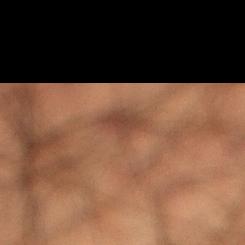Recorded during total-body skin imaging; not selected for excision or biopsy.
The patient is a male aged 48 to 52.
Imaged with cross-polarized lighting.
The lesion's longest dimension is about 3 mm.
Cropped from a whole-body photographic skin survey; the tile spans about 15 mm.
The lesion is on the right lower leg.